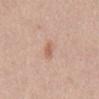Clinical impression:
Imaged during a routine full-body skin examination; the lesion was not biopsied and no histopathology is available.
Background:
A female patient, in their 40s. On the mid back. This image is a 15 mm lesion crop taken from a total-body photograph.